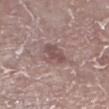Part of a total-body skin-imaging series; this lesion was reviewed on a skin check and was not flagged for biopsy.
A 15 mm close-up tile from a total-body photography series done for melanoma screening.
Captured under white-light illumination.
From the leg.
The patient is a female aged 73 to 77.